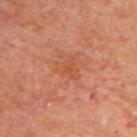follow-up — no biopsy performed (imaged during a skin exam)
TBP lesion metrics — a lesion area of about 4 mm², a shape eccentricity near 0.65, and two-axis asymmetry of about 0.55; an average lesion color of about L≈51 a*≈29 b*≈36 (CIELAB), roughly 5 lightness units darker than nearby skin, and a normalized lesion–skin contrast near 5; internal color variation of about 2 on a 0–10 scale and a peripheral color-asymmetry measure near 0.5; an automated nevus-likeness rating near 0 out of 100
image — total-body-photography crop, ~15 mm field of view
tile lighting — cross-polarized illumination
anatomic site — the upper back
subject — male, roughly 70 years of age